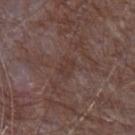This lesion was catalogued during total-body skin photography and was not selected for biopsy. Measured at roughly 2.5 mm in maximum diameter. A male subject, roughly 65 years of age. A roughly 15 mm field-of-view crop from a total-body skin photograph. From the left forearm.